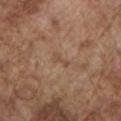Part of a total-body skin-imaging series; this lesion was reviewed on a skin check and was not flagged for biopsy.
A male subject roughly 75 years of age.
A 15 mm close-up extracted from a 3D total-body photography capture.
The lesion is on the left upper arm.
Imaged with white-light lighting.
Automated tile analysis of the lesion measured a footprint of about 2.5 mm², a shape eccentricity near 0.9, and a symmetry-axis asymmetry near 0.5. And it measured a lesion color around L≈49 a*≈18 b*≈29 in CIELAB and roughly 6 lightness units darker than nearby skin.